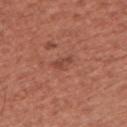{
  "biopsy_status": "not biopsied; imaged during a skin examination",
  "lesion_size": {
    "long_diameter_mm_approx": 3.0
  },
  "image": {
    "source": "total-body photography crop",
    "field_of_view_mm": 15
  },
  "site": "right upper arm",
  "lighting": "white-light",
  "patient": {
    "sex": "male",
    "age_approx": 45
  },
  "automated_metrics": {
    "area_mm2_approx": 3.0,
    "eccentricity": 0.9,
    "shape_asymmetry": 0.3,
    "cielab_L": 46,
    "cielab_a": 27,
    "cielab_b": 29,
    "vs_skin_darker_L": 7.0,
    "vs_skin_contrast_norm": 5.5,
    "nevus_likeness_0_100": 5,
    "lesion_detection_confidence_0_100": 100
  }
}